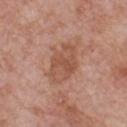<case>
<site>chest</site>
<lesion_size>
  <long_diameter_mm_approx>5.5</long_diameter_mm_approx>
</lesion_size>
<image>
  <source>total-body photography crop</source>
  <field_of_view_mm>15</field_of_view_mm>
</image>
<automated_metrics>
  <eccentricity>0.8</eccentricity>
  <cielab_L>53</cielab_L>
  <cielab_a>22</cielab_a>
  <cielab_b>30</cielab_b>
  <vs_skin_darker_L>8.0</vs_skin_darker_L>
</automated_metrics>
<patient>
  <sex>male</sex>
  <age_approx>60</age_approx>
</patient>
</case>